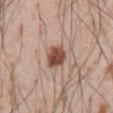| feature | finding |
|---|---|
| follow-up | total-body-photography surveillance lesion; no biopsy |
| patient | male, about 55 years old |
| automated metrics | an average lesion color of about L≈48 a*≈21 b*≈28 (CIELAB) and a lesion-to-skin contrast of about 11 (normalized; higher = more distinct); a nevus-likeness score of about 95/100 and lesion-presence confidence of about 100/100 |
| lesion size | ≈2.5 mm |
| illumination | white-light illumination |
| location | the abdomen |
| image | ~15 mm crop, total-body skin-cancer survey |